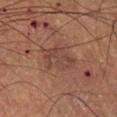This lesion was catalogued during total-body skin photography and was not selected for biopsy.
Located on the right lower leg.
A lesion tile, about 15 mm wide, cut from a 3D total-body photograph.
The tile uses cross-polarized illumination.
A male subject aged 53 to 57.